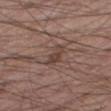<case>
  <biopsy_status>not biopsied; imaged during a skin examination</biopsy_status>
  <lighting>white-light</lighting>
  <automated_metrics>
    <eccentricity>0.9</eccentricity>
    <shape_asymmetry>0.35</shape_asymmetry>
    <vs_skin_darker_L>8.0</vs_skin_darker_L>
    <vs_skin_contrast_norm>6.5</vs_skin_contrast_norm>
    <nevus_likeness_0_100>0</nevus_likeness_0_100>
    <lesion_detection_confidence_0_100>95</lesion_detection_confidence_0_100>
  </automated_metrics>
  <site>right thigh</site>
  <lesion_size>
    <long_diameter_mm_approx>3.5</long_diameter_mm_approx>
  </lesion_size>
  <patient>
    <sex>male</sex>
    <age_approx>60</age_approx>
  </patient>
  <image>
    <source>total-body photography crop</source>
    <field_of_view_mm>15</field_of_view_mm>
  </image>
</case>